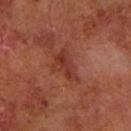Case summary:
* follow-up: catalogued during a skin exam; not biopsied
* location: the arm
* subject: male, approximately 60 years of age
* image source: total-body-photography crop, ~15 mm field of view